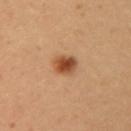Assessment: Part of a total-body skin-imaging series; this lesion was reviewed on a skin check and was not flagged for biopsy. Image and clinical context: This image is a 15 mm lesion crop taken from a total-body photograph. The subject is a female aged approximately 25. From the right upper arm.